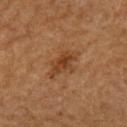– notes: no biopsy performed (imaged during a skin exam)
– image source: total-body-photography crop, ~15 mm field of view
– anatomic site: the upper back
– TBP lesion metrics: a mean CIELAB color near L≈37 a*≈20 b*≈32 and a normalized lesion–skin contrast near 7; a border-irregularity index near 4.5/10, internal color variation of about 3.5 on a 0–10 scale, and peripheral color asymmetry of about 1; an automated nevus-likeness rating near 35 out of 100 and a lesion-detection confidence of about 100/100
– lesion diameter: ≈4.5 mm
– patient: male, aged 63 to 67
– illumination: cross-polarized